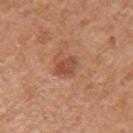<lesion>
  <biopsy_status>not biopsied; imaged during a skin examination</biopsy_status>
  <site>arm</site>
  <patient>
    <sex>male</sex>
    <age_approx>65</age_approx>
  </patient>
  <lesion_size>
    <long_diameter_mm_approx>3.0</long_diameter_mm_approx>
  </lesion_size>
  <image>
    <source>total-body photography crop</source>
    <field_of_view_mm>15</field_of_view_mm>
  </image>
</lesion>